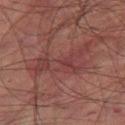follow-up: imaged on a skin check; not biopsied | acquisition: ~15 mm tile from a whole-body skin photo | location: the right thigh | subject: male, approximately 75 years of age.